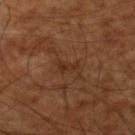Q: Was a biopsy performed?
A: no biopsy performed (imaged during a skin exam)
Q: What are the patient's age and sex?
A: male, aged around 60
Q: What is the anatomic site?
A: the right lower leg
Q: How was this image acquired?
A: ~15 mm crop, total-body skin-cancer survey